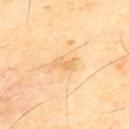workup — no biopsy performed (imaged during a skin exam) | subject — male, aged 63–67 | image — total-body-photography crop, ~15 mm field of view | body site — the upper back.